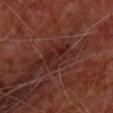  biopsy_status: not biopsied; imaged during a skin examination
  image:
    source: total-body photography crop
    field_of_view_mm: 15
  site: front of the torso
  lighting: cross-polarized
  patient:
    sex: male
    age_approx: 65
  automated_metrics:
    cielab_L: 21
    cielab_a: 21
    cielab_b: 20
    vs_skin_darker_L: 6.0
    vs_skin_contrast_norm: 7.0
    color_variation_0_10: 3.0
    peripheral_color_asymmetry: 1.0